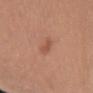<lesion>
  <biopsy_status>not biopsied; imaged during a skin examination</biopsy_status>
  <automated_metrics>
    <nevus_likeness_0_100>35</nevus_likeness_0_100>
    <lesion_detection_confidence_0_100>100</lesion_detection_confidence_0_100>
  </automated_metrics>
  <patient>
    <sex>female</sex>
    <age_approx>70</age_approx>
  </patient>
  <image>
    <source>total-body photography crop</source>
    <field_of_view_mm>15</field_of_view_mm>
  </image>
  <lesion_size>
    <long_diameter_mm_approx>2.5</long_diameter_mm_approx>
  </lesion_size>
  <site>upper back</site>
  <lighting>white-light</lighting>
</lesion>